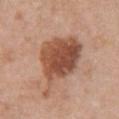workup: catalogued during a skin exam; not biopsied | TBP lesion metrics: two-axis asymmetry of about 0.35; a lesion–skin lightness drop of about 15 and a lesion-to-skin contrast of about 10 (normalized; higher = more distinct) | image: ~15 mm crop, total-body skin-cancer survey | lighting: white-light | body site: the front of the torso | patient: female, in their 60s | diameter: ~9 mm (longest diameter).